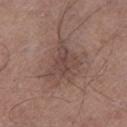biopsy status — catalogued during a skin exam; not biopsied
site — the left lower leg
subject — male, approximately 70 years of age
lesion size — ~5 mm (longest diameter)
tile lighting — white-light
image — total-body-photography crop, ~15 mm field of view